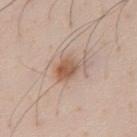Impression:
No biopsy was performed on this lesion — it was imaged during a full skin examination and was not determined to be concerning.
Acquisition and patient details:
Located on the chest. A roughly 15 mm field-of-view crop from a total-body skin photograph. Captured under white-light illumination. Approximately 4 mm at its widest. A male patient aged 58–62. An algorithmic analysis of the crop reported a border-irregularity index near 3.5/10, a within-lesion color-variation index near 4.5/10, and radial color variation of about 1.5.